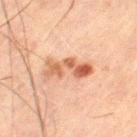This lesion was catalogued during total-body skin photography and was not selected for biopsy. Automated image analysis of the tile measured a lesion area of about 8.5 mm², a shape eccentricity near 0.95, and a symmetry-axis asymmetry near 0.45. And it measured an average lesion color of about L≈46 a*≈20 b*≈29 (CIELAB), roughly 11 lightness units darker than nearby skin, and a normalized lesion–skin contrast near 8.5. And it measured border irregularity of about 7.5 on a 0–10 scale, a within-lesion color-variation index near 8/10, and a peripheral color-asymmetry measure near 2.5. Located on the left thigh. A male patient, roughly 60 years of age. A 15 mm close-up tile from a total-body photography series done for melanoma screening. Approximately 5.5 mm at its widest.